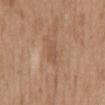| feature | finding |
|---|---|
| biopsy status | catalogued during a skin exam; not biopsied |
| lesion diameter | about 3 mm |
| image source | total-body-photography crop, ~15 mm field of view |
| patient | female, roughly 40 years of age |
| illumination | white-light illumination |
| TBP lesion metrics | an area of roughly 3 mm², a shape eccentricity near 0.9, and two-axis asymmetry of about 0.3 |
| site | the mid back |